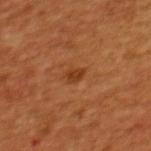Clinical impression: Imaged during a routine full-body skin examination; the lesion was not biopsied and no histopathology is available. Image and clinical context: The subject is a male aged 43–47. Longest diameter approximately 2 mm. The tile uses cross-polarized illumination. From the upper back. A region of skin cropped from a whole-body photographic capture, roughly 15 mm wide.